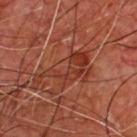follow-up = catalogued during a skin exam; not biopsied
lighting = cross-polarized
site = the chest
diameter = about 6.5 mm
subject = male, aged around 65
imaging modality = total-body-photography crop, ~15 mm field of view